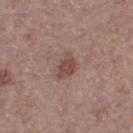The lesion was photographed on a routine skin check and not biopsied; there is no pathology result. The lesion's longest dimension is about 3 mm. The subject is a female aged approximately 40. Located on the right thigh. The tile uses white-light illumination. A close-up tile cropped from a whole-body skin photograph, about 15 mm across. Automated image analysis of the tile measured an area of roughly 5 mm² and an outline eccentricity of about 0.65 (0 = round, 1 = elongated). The software also gave an average lesion color of about L≈46 a*≈20 b*≈21 (CIELAB), a lesion–skin lightness drop of about 9, and a normalized lesion–skin contrast near 7. The software also gave a lesion-detection confidence of about 100/100.